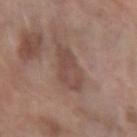<lesion>
<biopsy_status>not biopsied; imaged during a skin examination</biopsy_status>
<site>left forearm</site>
<patient>
  <sex>female</sex>
  <age_approx>60</age_approx>
</patient>
<image>
  <source>total-body photography crop</source>
  <field_of_view_mm>15</field_of_view_mm>
</image>
</lesion>